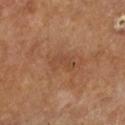workup: total-body-photography surveillance lesion; no biopsy | lesion size: about 3.5 mm | anatomic site: the left lower leg | image source: ~15 mm crop, total-body skin-cancer survey | subject: male, roughly 65 years of age | lighting: cross-polarized | image-analysis metrics: border irregularity of about 4 on a 0–10 scale, a within-lesion color-variation index near 1/10, and radial color variation of about 0.5; a nevus-likeness score of about 0/100 and a detector confidence of about 100 out of 100 that the crop contains a lesion.